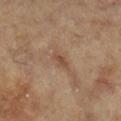Q: Was this lesion biopsied?
A: no biopsy performed (imaged during a skin exam)
Q: What are the patient's age and sex?
A: female, in their mid- to late 70s
Q: What is the imaging modality?
A: ~15 mm tile from a whole-body skin photo
Q: What is the anatomic site?
A: the leg